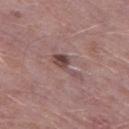Part of a total-body skin-imaging series; this lesion was reviewed on a skin check and was not flagged for biopsy.
The tile uses white-light illumination.
The lesion is located on the left thigh.
Measured at roughly 4.5 mm in maximum diameter.
Cropped from a whole-body photographic skin survey; the tile spans about 15 mm.
The patient is a male in their 50s.